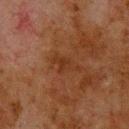workup: catalogued during a skin exam; not biopsied | anatomic site: the upper back | subject: male, aged approximately 80 | image source: ~15 mm crop, total-body skin-cancer survey | illumination: cross-polarized illumination | image-analysis metrics: an eccentricity of roughly 0.9 and two-axis asymmetry of about 0.45; a lesion color around L≈26 a*≈19 b*≈27 in CIELAB and a normalized border contrast of about 5.5; a border-irregularity index near 6.5/10, internal color variation of about 1 on a 0–10 scale, and peripheral color asymmetry of about 0.5; an automated nevus-likeness rating near 0 out of 100 and a detector confidence of about 100 out of 100 that the crop contains a lesion | lesion diameter: about 3.5 mm.